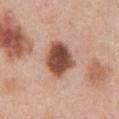No biopsy was performed on this lesion — it was imaged during a full skin examination and was not determined to be concerning.
Located on the abdomen.
The subject is a male aged around 70.
This is a white-light tile.
A lesion tile, about 15 mm wide, cut from a 3D total-body photograph.